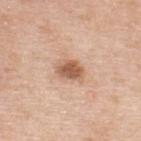Imaged during a routine full-body skin examination; the lesion was not biopsied and no histopathology is available. On the upper back. A region of skin cropped from a whole-body photographic capture, roughly 15 mm wide. The subject is a male approximately 50 years of age.